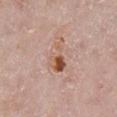biopsy_status: not biopsied; imaged during a skin examination
lighting: white-light
patient:
  sex: female
  age_approx: 65
automated_metrics:
  eccentricity: 0.8
  cielab_L: 55
  cielab_a: 21
  cielab_b: 31
  vs_skin_darker_L: 11.0
  vs_skin_contrast_norm: 8.5
  border_irregularity_0_10: 7.0
  color_variation_0_10: 10.0
  peripheral_color_asymmetry: 4.0
  nevus_likeness_0_100: 75
  lesion_detection_confidence_0_100: 100
site: right lower leg
lesion_size:
  long_diameter_mm_approx: 4.0
image:
  source: total-body photography crop
  field_of_view_mm: 15